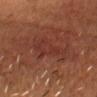The lesion was tiled from a total-body skin photograph and was not biopsied.
The lesion is located on the head or neck.
Cropped from a whole-body photographic skin survey; the tile spans about 15 mm.
Measured at roughly 5 mm in maximum diameter.
The subject is a male aged approximately 55.
The tile uses cross-polarized illumination.